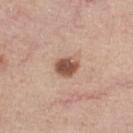The lesion was tiled from a total-body skin photograph and was not biopsied.
The lesion is on the right thigh.
A female patient aged approximately 50.
A close-up tile cropped from a whole-body skin photograph, about 15 mm across.
This is a white-light tile.